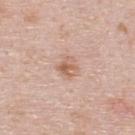Context: A male subject, aged around 40. On the upper back. A 15 mm crop from a total-body photograph taken for skin-cancer surveillance. The lesion's longest dimension is about 2 mm.